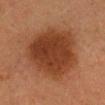Imaged during a routine full-body skin examination; the lesion was not biopsied and no histopathology is available. Approximately 7.5 mm at its widest. Imaged with cross-polarized lighting. The lesion-visualizer software estimated a classifier nevus-likeness of about 100/100 and a lesion-detection confidence of about 100/100. The lesion is on the head or neck. A 15 mm crop from a total-body photograph taken for skin-cancer surveillance. A female patient, aged around 50.